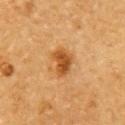Clinical impression: Part of a total-body skin-imaging series; this lesion was reviewed on a skin check and was not flagged for biopsy. Acquisition and patient details: A 15 mm close-up tile from a total-body photography series done for melanoma screening. The lesion is on the arm. The patient is a male in their mid-80s or older. Imaged with cross-polarized lighting. Measured at roughly 3.5 mm in maximum diameter.